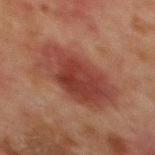| key | value |
|---|---|
| notes | catalogued during a skin exam; not biopsied |
| lesion diameter | ~8 mm (longest diameter) |
| subject | male, aged around 65 |
| image | total-body-photography crop, ~15 mm field of view |
| tile lighting | cross-polarized illumination |
| image-analysis metrics | an area of roughly 30 mm², a shape eccentricity near 0.8, and two-axis asymmetry of about 0.15; a lesion color around L≈32 a*≈22 b*≈23 in CIELAB, a lesion–skin lightness drop of about 8, and a lesion-to-skin contrast of about 8 (normalized; higher = more distinct) |
| location | the chest |